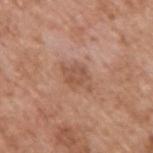No biopsy was performed on this lesion — it was imaged during a full skin examination and was not determined to be concerning.
Approximately 4 mm at its widest.
A male subject, approximately 65 years of age.
This image is a 15 mm lesion crop taken from a total-body photograph.
On the upper back.